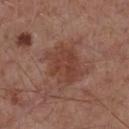Captured during whole-body skin photography for melanoma surveillance; the lesion was not biopsied. The lesion-visualizer software estimated a footprint of about 14 mm² and two-axis asymmetry of about 0.25. The analysis additionally found a color-variation rating of about 3/10 and a peripheral color-asymmetry measure near 1. A male patient aged approximately 55. A 15 mm close-up tile from a total-body photography series done for melanoma screening. Located on the chest. The lesion's longest dimension is about 4.5 mm.